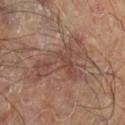{"biopsy_status": "not biopsied; imaged during a skin examination", "lesion_size": {"long_diameter_mm_approx": 8.0}, "image": {"source": "total-body photography crop", "field_of_view_mm": 15}, "lighting": "cross-polarized", "site": "right lower leg", "patient": {"sex": "male", "age_approx": 70}}